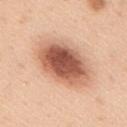Findings:
• notes: imaged on a skin check; not biopsied
• subject: male, aged 33 to 37
• tile lighting: white-light illumination
• image: total-body-photography crop, ~15 mm field of view
• lesion size: about 7 mm
• anatomic site: the mid back